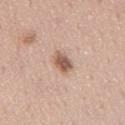  biopsy_status: not biopsied; imaged during a skin examination
  image:
    source: total-body photography crop
    field_of_view_mm: 15
  lighting: white-light
  site: mid back
  patient:
    sex: male
    age_approx: 60
  lesion_size:
    long_diameter_mm_approx: 3.5